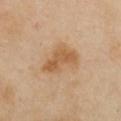No biopsy was performed on this lesion — it was imaged during a full skin examination and was not determined to be concerning. A region of skin cropped from a whole-body photographic capture, roughly 15 mm wide. The tile uses cross-polarized illumination. Located on the chest. The patient is a female aged around 40.